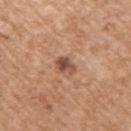Context:
Imaged with white-light lighting. The lesion is on the arm. The total-body-photography lesion software estimated an average lesion color of about L≈50 a*≈21 b*≈29 (CIELAB), a lesion–skin lightness drop of about 13, and a normalized border contrast of about 9. The recorded lesion diameter is about 2.5 mm. A male subject, aged around 60. A 15 mm close-up tile from a total-body photography series done for melanoma screening.